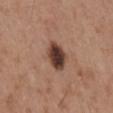Impression:
Part of a total-body skin-imaging series; this lesion was reviewed on a skin check and was not flagged for biopsy.
Acquisition and patient details:
A male subject about 55 years old. Captured under white-light illumination. Located on the mid back. Cropped from a whole-body photographic skin survey; the tile spans about 15 mm. Measured at roughly 3.5 mm in maximum diameter.